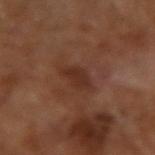Recorded during total-body skin imaging; not selected for excision or biopsy.
This image is a 15 mm lesion crop taken from a total-body photograph.
An algorithmic analysis of the crop reported a footprint of about 5.5 mm², an outline eccentricity of about 0.9 (0 = round, 1 = elongated), and two-axis asymmetry of about 0.3. It also reported an average lesion color of about L≈30 a*≈20 b*≈25 (CIELAB), a lesion–skin lightness drop of about 7, and a normalized border contrast of about 7.
The patient is a male aged 63 to 67.
Approximately 4 mm at its widest.